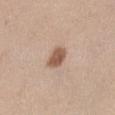{
  "biopsy_status": "not biopsied; imaged during a skin examination",
  "site": "right thigh",
  "automated_metrics": {
    "area_mm2_approx": 5.0,
    "eccentricity": 0.6,
    "shape_asymmetry": 0.25,
    "cielab_L": 56,
    "cielab_a": 19,
    "cielab_b": 29,
    "vs_skin_contrast_norm": 9.0,
    "border_irregularity_0_10": 2.0,
    "color_variation_0_10": 2.5,
    "peripheral_color_asymmetry": 1.0,
    "nevus_likeness_0_100": 95
  },
  "lesion_size": {
    "long_diameter_mm_approx": 2.5
  },
  "image": {
    "source": "total-body photography crop",
    "field_of_view_mm": 15
  },
  "patient": {
    "sex": "female",
    "age_approx": 40
  },
  "lighting": "white-light"
}